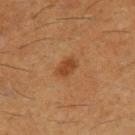The patient is a male aged approximately 60.
On the right thigh.
Cropped from a total-body skin-imaging series; the visible field is about 15 mm.